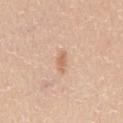workup — imaged on a skin check; not biopsied.